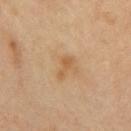  biopsy_status: not biopsied; imaged during a skin examination
  site: mid back
  lighting: cross-polarized
  image:
    source: total-body photography crop
    field_of_view_mm: 15
  lesion_size:
    long_diameter_mm_approx: 3.0
  patient:
    sex: male
    age_approx: 55